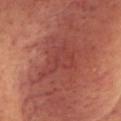Captured during whole-body skin photography for melanoma surveillance; the lesion was not biopsied.
The lesion is on the head or neck.
A 15 mm crop from a total-body photograph taken for skin-cancer surveillance.
A female patient, aged approximately 55.